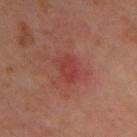Recorded during total-body skin imaging; not selected for excision or biopsy.
A 15 mm close-up tile from a total-body photography series done for melanoma screening.
The patient is a male aged 38 to 42.
Longest diameter approximately 3 mm.
On the chest.
Imaged with cross-polarized lighting.